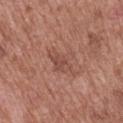A 15 mm close-up extracted from a 3D total-body photography capture. A male patient aged 48–52. The lesion's longest dimension is about 4 mm. This is a white-light tile. On the mid back. Automated image analysis of the tile measured a border-irregularity rating of about 5/10, a within-lesion color-variation index near 2.5/10, and peripheral color asymmetry of about 0.5.